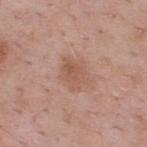The lesion was photographed on a routine skin check and not biopsied; there is no pathology result. Longest diameter approximately 3.5 mm. A male patient in their mid- to late 50s. A close-up tile cropped from a whole-body skin photograph, about 15 mm across. The lesion is on the upper back. Captured under white-light illumination.